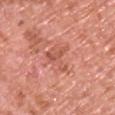workup: total-body-photography surveillance lesion; no biopsy
subject: male, about 70 years old
acquisition: ~15 mm crop, total-body skin-cancer survey
body site: the front of the torso